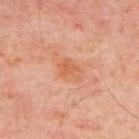Image and clinical context:
Located on the upper back. A patient in their mid-50s. Longest diameter approximately 3 mm. This image is a 15 mm lesion crop taken from a total-body photograph.